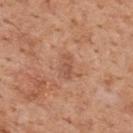This lesion was catalogued during total-body skin photography and was not selected for biopsy.
Automated image analysis of the tile measured a lesion area of about 4.5 mm², an outline eccentricity of about 0.85 (0 = round, 1 = elongated), and a symmetry-axis asymmetry near 0.25. The analysis additionally found a nevus-likeness score of about 0/100 and lesion-presence confidence of about 100/100.
Imaged with white-light lighting.
A 15 mm close-up tile from a total-body photography series done for melanoma screening.
From the upper back.
A male subject aged approximately 60.
Approximately 3 mm at its widest.